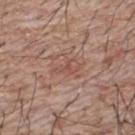subject = male, aged approximately 65
site = the upper back
image source = ~15 mm tile from a whole-body skin photo